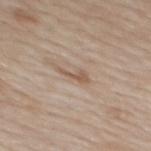{"biopsy_status": "not biopsied; imaged during a skin examination", "site": "mid back", "patient": {"sex": "male", "age_approx": 60}, "image": {"source": "total-body photography crop", "field_of_view_mm": 15}}